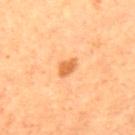Clinical impression: Part of a total-body skin-imaging series; this lesion was reviewed on a skin check and was not flagged for biopsy. Context: The subject is a female roughly 55 years of age. The total-body-photography lesion software estimated a lesion area of about 3 mm², a shape eccentricity near 0.8, and a shape-asymmetry score of about 0.3 (0 = symmetric). And it measured roughly 12 lightness units darker than nearby skin and a normalized lesion–skin contrast near 8. A region of skin cropped from a whole-body photographic capture, roughly 15 mm wide. The lesion is on the upper back.